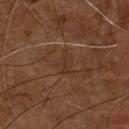No biopsy was performed on this lesion — it was imaged during a full skin examination and was not determined to be concerning.
This image is a 15 mm lesion crop taken from a total-body photograph.
Located on the front of the torso.
Imaged with cross-polarized lighting.
A male patient approximately 55 years of age.
The lesion-visualizer software estimated an area of roughly 4.5 mm², an eccentricity of roughly 0.55, and a symmetry-axis asymmetry near 0.4. And it measured a mean CIELAB color near L≈29 a*≈16 b*≈26, roughly 4 lightness units darker than nearby skin, and a normalized lesion–skin contrast near 4.5. And it measured a border-irregularity rating of about 4/10, internal color variation of about 1 on a 0–10 scale, and radial color variation of about 0.5.